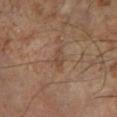Cropped from a total-body skin-imaging series; the visible field is about 15 mm.
Captured under cross-polarized illumination.
A male subject, roughly 60 years of age.
From the left lower leg.